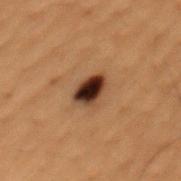Impression: Captured during whole-body skin photography for melanoma surveillance; the lesion was not biopsied. Acquisition and patient details: A male patient aged approximately 50. A roughly 15 mm field-of-view crop from a total-body skin photograph. The lesion is on the back.